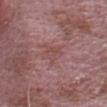Q: Was a biopsy performed?
A: catalogued during a skin exam; not biopsied
Q: Patient demographics?
A: male, about 65 years old
Q: Lesion size?
A: about 3 mm
Q: How was the tile lit?
A: white-light illumination
Q: Where on the body is the lesion?
A: the head or neck
Q: What kind of image is this?
A: total-body-photography crop, ~15 mm field of view